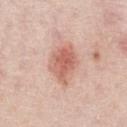Assessment:
No biopsy was performed on this lesion — it was imaged during a full skin examination and was not determined to be concerning.
Context:
Located on the chest. A 15 mm close-up tile from a total-body photography series done for melanoma screening. This is a white-light tile. A male subject in their 60s. The recorded lesion diameter is about 4.5 mm.